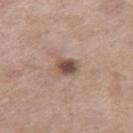The lesion was tiled from a total-body skin photograph and was not biopsied. The patient is a female in their mid- to late 50s. This is a white-light tile. From the right thigh. A 15 mm close-up tile from a total-body photography series done for melanoma screening.